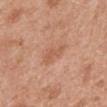Q: Is there a histopathology result?
A: imaged on a skin check; not biopsied
Q: Patient demographics?
A: female, aged around 40
Q: What is the imaging modality?
A: total-body-photography crop, ~15 mm field of view
Q: Where on the body is the lesion?
A: the mid back